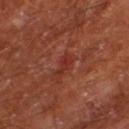Located on the left thigh. A male subject, aged approximately 65. A 15 mm close-up tile from a total-body photography series done for melanoma screening.